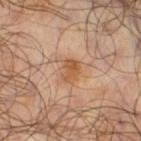site: the right thigh
TBP lesion metrics: an area of roughly 5 mm², an eccentricity of roughly 0.75, and a shape-asymmetry score of about 0.25 (0 = symmetric); an automated nevus-likeness rating near 70 out of 100
illumination: cross-polarized illumination
subject: male, roughly 65 years of age
image source: total-body-photography crop, ~15 mm field of view
diameter: ~3 mm (longest diameter)